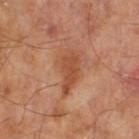follow-up = total-body-photography surveillance lesion; no biopsy
lighting = cross-polarized
image = ~15 mm tile from a whole-body skin photo
image-analysis metrics = an eccentricity of roughly 0.9 and two-axis asymmetry of about 0.4; a lesion color around L≈48 a*≈25 b*≈34 in CIELAB, a lesion–skin lightness drop of about 9, and a normalized border contrast of about 7; a within-lesion color-variation index near 2/10 and a peripheral color-asymmetry measure near 1
patient = male, in their mid- to late 60s